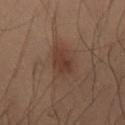Clinical impression: Recorded during total-body skin imaging; not selected for excision or biopsy. Acquisition and patient details: About 3.5 mm across. Imaged with cross-polarized lighting. An algorithmic analysis of the crop reported an area of roughly 6 mm², an outline eccentricity of about 0.8 (0 = round, 1 = elongated), and a shape-asymmetry score of about 0.3 (0 = symmetric). The software also gave a lesion color around L≈28 a*≈15 b*≈21 in CIELAB, a lesion–skin lightness drop of about 6, and a lesion-to-skin contrast of about 7 (normalized; higher = more distinct). It also reported a border-irregularity rating of about 3/10 and a color-variation rating of about 2/10. On the right thigh. This image is a 15 mm lesion crop taken from a total-body photograph. A male subject aged 53–57.